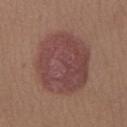Q: Is there a histopathology result?
A: total-body-photography surveillance lesion; no biopsy
Q: Patient demographics?
A: male, aged approximately 30
Q: How large is the lesion?
A: ≈8.5 mm
Q: How was this image acquired?
A: 15 mm crop, total-body photography
Q: How was the tile lit?
A: white-light
Q: Where on the body is the lesion?
A: the right lower leg